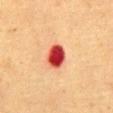biopsy status: imaged on a skin check; not biopsied | image source: total-body-photography crop, ~15 mm field of view | automated metrics: an area of roughly 7 mm² and two-axis asymmetry of about 0.1; an average lesion color of about L≈41 a*≈38 b*≈32 (CIELAB) and roughly 21 lightness units darker than nearby skin; a border-irregularity index near 1/10 and peripheral color asymmetry of about 1.5 | lesion size: about 3.5 mm | subject: female, aged around 55 | anatomic site: the abdomen | lighting: cross-polarized illumination.